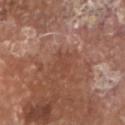Context: Measured at roughly 2.5 mm in maximum diameter. The patient is a male aged approximately 80. A 15 mm crop from a total-body photograph taken for skin-cancer surveillance. Located on the head or neck.